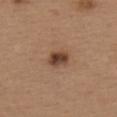workup = no biopsy performed (imaged during a skin exam) | illumination = white-light illumination | automated lesion analysis = border irregularity of about 1.5 on a 0–10 scale, a color-variation rating of about 5/10, and radial color variation of about 1.5 | size = about 3 mm | patient = female, approximately 40 years of age | acquisition = 15 mm crop, total-body photography | anatomic site = the upper back.